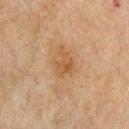Assessment: Recorded during total-body skin imaging; not selected for excision or biopsy. Context: Measured at roughly 3 mm in maximum diameter. The tile uses cross-polarized illumination. From the front of the torso. An algorithmic analysis of the crop reported a lesion color around L≈45 a*≈17 b*≈32 in CIELAB, a lesion–skin lightness drop of about 7, and a normalized border contrast of about 6. It also reported a border-irregularity rating of about 4/10, a within-lesion color-variation index near 3/10, and peripheral color asymmetry of about 1. The analysis additionally found a nevus-likeness score of about 5/100 and a lesion-detection confidence of about 100/100. A male subject, aged 68 to 72. Cropped from a whole-body photographic skin survey; the tile spans about 15 mm.